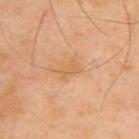The lesion was tiled from a total-body skin photograph and was not biopsied. A roughly 15 mm field-of-view crop from a total-body skin photograph. A male subject aged 38 to 42. The lesion is on the upper back.